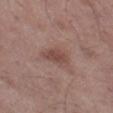Impression:
Imaged during a routine full-body skin examination; the lesion was not biopsied and no histopathology is available.
Acquisition and patient details:
The lesion is located on the leg. A male patient, aged 48 to 52. A 15 mm crop from a total-body photograph taken for skin-cancer surveillance. The tile uses white-light illumination.